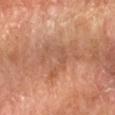A 15 mm close-up extracted from a 3D total-body photography capture. The lesion is on the left forearm. This is a cross-polarized tile. A male patient, in their 60s. Measured at roughly 7 mm in maximum diameter.